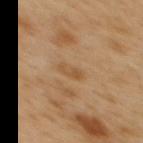The recorded lesion diameter is about 2.5 mm.
An algorithmic analysis of the crop reported a classifier nevus-likeness of about 0/100 and a lesion-detection confidence of about 100/100.
A region of skin cropped from a whole-body photographic capture, roughly 15 mm wide.
From the mid back.
A male patient, aged approximately 50.
Captured under cross-polarized illumination.